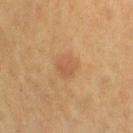notes: total-body-photography surveillance lesion; no biopsy
location: the right lower leg
image: ~15 mm tile from a whole-body skin photo
patient: female, roughly 50 years of age